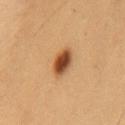  biopsy_status: not biopsied; imaged during a skin examination
  lighting: cross-polarized
  patient:
    sex: male
    age_approx: 55
  lesion_size:
    long_diameter_mm_approx: 3.5
  image:
    source: total-body photography crop
    field_of_view_mm: 15
  site: chest